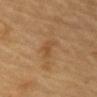The tile uses cross-polarized illumination. Cropped from a whole-body photographic skin survey; the tile spans about 15 mm. Approximately 3 mm at its widest. Located on the front of the torso. The subject is a male about 85 years old or older.